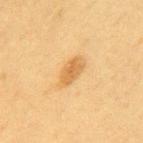notes: imaged on a skin check; not biopsied.